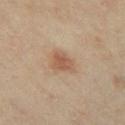notes: total-body-photography surveillance lesion; no biopsy | illumination: cross-polarized | patient: female, aged around 35 | diameter: ≈2.5 mm | anatomic site: the abdomen | image source: total-body-photography crop, ~15 mm field of view.